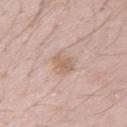Q: Was a biopsy performed?
A: no biopsy performed (imaged during a skin exam)